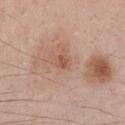Q: Is there a histopathology result?
A: imaged on a skin check; not biopsied
Q: Patient demographics?
A: male, in their mid-30s
Q: Lesion size?
A: ~1.5 mm (longest diameter)
Q: What did automated image analysis measure?
A: an area of roughly 1.5 mm² and a shape-asymmetry score of about 0.15 (0 = symmetric); about 9 CIELAB-L* units darker than the surrounding skin and a normalized border contrast of about 6.5; a classifier nevus-likeness of about 5/100 and a detector confidence of about 100 out of 100 that the crop contains a lesion
Q: What lighting was used for the tile?
A: white-light
Q: How was this image acquired?
A: 15 mm crop, total-body photography
Q: Where on the body is the lesion?
A: the chest